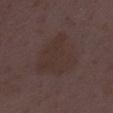follow-up: catalogued during a skin exam; not biopsied
lesion diameter: ≈6.5 mm
anatomic site: the leg
image source: 15 mm crop, total-body photography
illumination: white-light
subject: female, aged 28 to 32